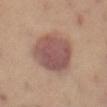Imaged during a routine full-body skin examination; the lesion was not biopsied and no histopathology is available.
A female subject in their mid-50s.
Approximately 6 mm at its widest.
The lesion-visualizer software estimated a mean CIELAB color near L≈52 a*≈21 b*≈22 and a normalized border contrast of about 9.
Located on the front of the torso.
A 15 mm crop from a total-body photograph taken for skin-cancer surveillance.
The tile uses cross-polarized illumination.